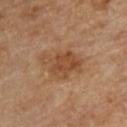Impression:
The lesion was photographed on a routine skin check and not biopsied; there is no pathology result.
Background:
From the upper back. Approximately 5 mm at its widest. Cropped from a whole-body photographic skin survey; the tile spans about 15 mm. A male patient about 85 years old or older. The tile uses cross-polarized illumination. An algorithmic analysis of the crop reported a border-irregularity rating of about 4/10, a within-lesion color-variation index near 3/10, and radial color variation of about 1. The analysis additionally found a classifier nevus-likeness of about 75/100 and lesion-presence confidence of about 100/100.